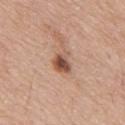A 15 mm close-up tile from a total-body photography series done for melanoma screening.
The lesion is located on the upper back.
The lesion-visualizer software estimated a lesion area of about 5.5 mm² and a shape eccentricity near 0.6. The analysis additionally found an average lesion color of about L≈53 a*≈21 b*≈29 (CIELAB), a lesion–skin lightness drop of about 14, and a lesion-to-skin contrast of about 9.5 (normalized; higher = more distinct).
Measured at roughly 3 mm in maximum diameter.
The patient is a male aged 73–77.
The tile uses white-light illumination.